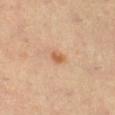Notes:
* patient · female, aged 43 to 47
* image source · total-body-photography crop, ~15 mm field of view
* site · the right lower leg